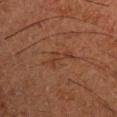No biopsy was performed on this lesion — it was imaged during a full skin examination and was not determined to be concerning. The lesion is located on the right upper arm. Captured under cross-polarized illumination. A 15 mm close-up tile from a total-body photography series done for melanoma screening. A male patient, aged approximately 50.